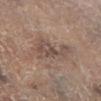This lesion was catalogued during total-body skin photography and was not selected for biopsy. The tile uses cross-polarized illumination. Cropped from a whole-body photographic skin survey; the tile spans about 15 mm. Automated tile analysis of the lesion measured a lesion area of about 11 mm² and an eccentricity of roughly 0.8. And it measured roughly 8 lightness units darker than nearby skin and a lesion-to-skin contrast of about 6.5 (normalized; higher = more distinct). It also reported an automated nevus-likeness rating near 0 out of 100 and a lesion-detection confidence of about 90/100. The lesion is on the left lower leg. The subject is a male in their mid- to late 60s.